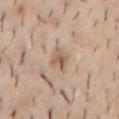<lesion>
<biopsy_status>not biopsied; imaged during a skin examination</biopsy_status>
<lesion_size>
  <long_diameter_mm_approx>3.0</long_diameter_mm_approx>
</lesion_size>
<lighting>white-light</lighting>
<image>
  <source>total-body photography crop</source>
  <field_of_view_mm>15</field_of_view_mm>
</image>
<site>chest</site>
<patient>
  <sex>male</sex>
  <age_approx>40</age_approx>
</patient>
</lesion>